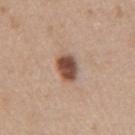This image is a 15 mm lesion crop taken from a total-body photograph.
The recorded lesion diameter is about 3 mm.
The subject is a female in their 40s.
The lesion is located on the right upper arm.
Automated tile analysis of the lesion measured a mean CIELAB color near L≈48 a*≈20 b*≈28 and about 17 CIELAB-L* units darker than the surrounding skin. The software also gave border irregularity of about 1.5 on a 0–10 scale, a within-lesion color-variation index near 4.5/10, and a peripheral color-asymmetry measure near 1.5.